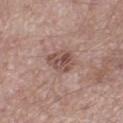Recorded during total-body skin imaging; not selected for excision or biopsy. A lesion tile, about 15 mm wide, cut from a 3D total-body photograph. A male subject, roughly 55 years of age. The lesion is located on the right thigh. Approximately 3 mm at its widest. This is a white-light tile.